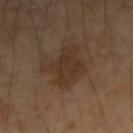Impression:
The lesion was photographed on a routine skin check and not biopsied; there is no pathology result.
Background:
The subject is a male aged approximately 45. This is a cross-polarized tile. A 15 mm close-up tile from a total-body photography series done for melanoma screening. On the right forearm. The total-body-photography lesion software estimated an area of roughly 13 mm². And it measured an average lesion color of about L≈31 a*≈15 b*≈25 (CIELAB) and about 7 CIELAB-L* units darker than the surrounding skin. The analysis additionally found an automated nevus-likeness rating near 25 out of 100.